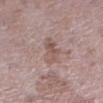Part of a total-body skin-imaging series; this lesion was reviewed on a skin check and was not flagged for biopsy.
Automated image analysis of the tile measured a border-irregularity index near 4.5/10 and radial color variation of about 1.5. It also reported an automated nevus-likeness rating near 0 out of 100 and lesion-presence confidence of about 100/100.
Longest diameter approximately 4 mm.
Captured under white-light illumination.
A 15 mm close-up tile from a total-body photography series done for melanoma screening.
On the left lower leg.
The subject is a female aged around 70.